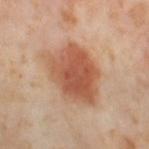workup: total-body-photography surveillance lesion; no biopsy
tile lighting: cross-polarized illumination
site: the leg
image: ~15 mm crop, total-body skin-cancer survey
diameter: ~7.5 mm (longest diameter)
automated metrics: a border-irregularity rating of about 2.5/10 and a peripheral color-asymmetry measure near 1.5; a classifier nevus-likeness of about 100/100
patient: female, approximately 55 years of age